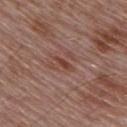Q: What is the anatomic site?
A: the upper back
Q: What lighting was used for the tile?
A: white-light illumination
Q: What is the lesion's diameter?
A: about 2.5 mm
Q: What is the imaging modality?
A: ~15 mm crop, total-body skin-cancer survey
Q: Patient demographics?
A: male, in their mid-50s